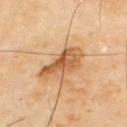tile lighting: cross-polarized | subject: male, roughly 65 years of age | anatomic site: the upper back | size: ~5.5 mm (longest diameter) | image-analysis metrics: an area of roughly 11 mm², an eccentricity of roughly 0.85, and a symmetry-axis asymmetry near 0.3; an average lesion color of about L≈60 a*≈22 b*≈40 (CIELAB), about 13 CIELAB-L* units darker than the surrounding skin, and a normalized lesion–skin contrast near 8; border irregularity of about 3.5 on a 0–10 scale and a peripheral color-asymmetry measure near 2; an automated nevus-likeness rating near 0 out of 100 and a lesion-detection confidence of about 100/100 | image: total-body-photography crop, ~15 mm field of view.